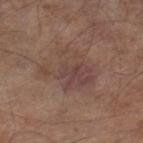{"biopsy_status": "not biopsied; imaged during a skin examination", "patient": {"sex": "male", "age_approx": 80}, "site": "right lower leg", "lesion_size": {"long_diameter_mm_approx": 5.5}, "lighting": "white-light", "automated_metrics": {"area_mm2_approx": 17.0, "eccentricity": 0.6, "shape_asymmetry": 0.4, "cielab_L": 43, "cielab_a": 18, "cielab_b": 22, "vs_skin_contrast_norm": 5.5}, "image": {"source": "total-body photography crop", "field_of_view_mm": 15}}